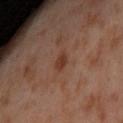Impression:
The lesion was photographed on a routine skin check and not biopsied; there is no pathology result.
Image and clinical context:
A roughly 15 mm field-of-view crop from a total-body skin photograph. The patient is a female aged around 55. On the left thigh. Measured at roughly 2.5 mm in maximum diameter. Captured under cross-polarized illumination.